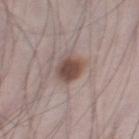The lesion-visualizer software estimated a nevus-likeness score of about 95/100.
Measured at roughly 3 mm in maximum diameter.
Imaged with white-light lighting.
Located on the right thigh.
A male subject, about 70 years old.
A lesion tile, about 15 mm wide, cut from a 3D total-body photograph.